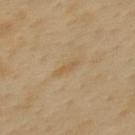Impression:
Recorded during total-body skin imaging; not selected for excision or biopsy.
Image and clinical context:
Longest diameter approximately 3 mm. The lesion-visualizer software estimated border irregularity of about 3.5 on a 0–10 scale, a within-lesion color-variation index near 0/10, and peripheral color asymmetry of about 0. And it measured an automated nevus-likeness rating near 0 out of 100. A female subject roughly 50 years of age. The lesion is on the upper back. Cropped from a total-body skin-imaging series; the visible field is about 15 mm.